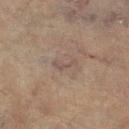Case summary:
- biopsy status · imaged on a skin check; not biopsied
- diameter · ≈3 mm
- body site · the leg
- patient · female, in their 80s
- tile lighting · cross-polarized illumination
- image · total-body-photography crop, ~15 mm field of view